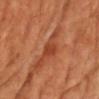workup = total-body-photography surveillance lesion; no biopsy
illumination = cross-polarized
size = ≈2.5 mm
automated lesion analysis = a detector confidence of about 100 out of 100 that the crop contains a lesion
image = ~15 mm tile from a whole-body skin photo
anatomic site = the chest
subject = male, roughly 65 years of age